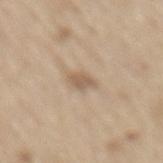The lesion was photographed on a routine skin check and not biopsied; there is no pathology result.
Cropped from a whole-body photographic skin survey; the tile spans about 15 mm.
On the mid back.
A male patient roughly 70 years of age.
An algorithmic analysis of the crop reported an area of roughly 3.5 mm², a shape eccentricity near 0.8, and a shape-asymmetry score of about 0.35 (0 = symmetric). It also reported a lesion color around L≈59 a*≈14 b*≈30 in CIELAB and a normalized border contrast of about 6.5. And it measured a border-irregularity rating of about 3.5/10, a within-lesion color-variation index near 1.5/10, and radial color variation of about 0.5.